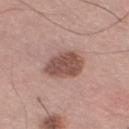The subject is a male aged approximately 70. The lesion is on the right thigh. A 15 mm close-up extracted from a 3D total-body photography capture.